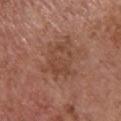{
  "biopsy_status": "not biopsied; imaged during a skin examination",
  "image": {
    "source": "total-body photography crop",
    "field_of_view_mm": 15
  },
  "patient": {
    "sex": "female",
    "age_approx": 65
  },
  "lighting": "white-light",
  "automated_metrics": {
    "peripheral_color_asymmetry": 1.0
  },
  "site": "upper back"
}